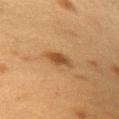biopsy status: no biopsy performed (imaged during a skin exam); diameter: about 2.5 mm; body site: the right upper arm; subject: female, approximately 40 years of age; image source: 15 mm crop, total-body photography; illumination: cross-polarized.